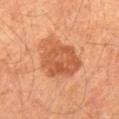notes = imaged on a skin check; not biopsied
subject = male, aged 63–67
location = the front of the torso
image = 15 mm crop, total-body photography